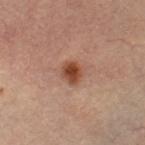workup — total-body-photography surveillance lesion; no biopsy | imaging modality — 15 mm crop, total-body photography | subject — female, in their mid-60s | site — the left thigh | tile lighting — cross-polarized.